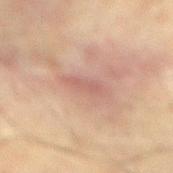{"biopsy_status": "not biopsied; imaged during a skin examination", "lighting": "cross-polarized", "image": {"source": "total-body photography crop", "field_of_view_mm": 15}, "patient": {"sex": "male", "age_approx": 75}, "site": "mid back", "lesion_size": {"long_diameter_mm_approx": 4.0}}